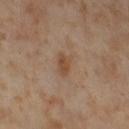No biopsy was performed on this lesion — it was imaged during a full skin examination and was not determined to be concerning. A female subject aged 53–57. Captured under cross-polarized illumination. A lesion tile, about 15 mm wide, cut from a 3D total-body photograph. The lesion-visualizer software estimated a border-irregularity rating of about 3/10, a color-variation rating of about 2/10, and radial color variation of about 0.5. And it measured an automated nevus-likeness rating near 10 out of 100 and a detector confidence of about 100 out of 100 that the crop contains a lesion. From the right thigh. Longest diameter approximately 3 mm.